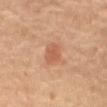The lesion was tiled from a total-body skin photograph and was not biopsied. A lesion tile, about 15 mm wide, cut from a 3D total-body photograph. The lesion is on the front of the torso. A female patient aged 58 to 62.